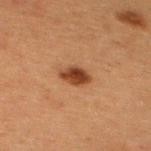The lesion was photographed on a routine skin check and not biopsied; there is no pathology result. A 15 mm crop from a total-body photograph taken for skin-cancer surveillance. A female subject about 40 years old. From the upper back. Longest diameter approximately 3 mm.